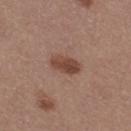Impression:
Imaged during a routine full-body skin examination; the lesion was not biopsied and no histopathology is available.
Background:
A 15 mm crop from a total-body photograph taken for skin-cancer surveillance. The lesion is on the right thigh. Captured under white-light illumination. A female subject aged approximately 35.